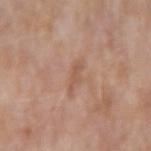lighting = white-light | lesion size = about 3 mm | patient = female, aged 68–72 | imaging modality = total-body-photography crop, ~15 mm field of view | site = the left forearm.